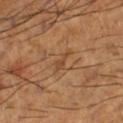Imaged during a routine full-body skin examination; the lesion was not biopsied and no histopathology is available.
Measured at roughly 2.5 mm in maximum diameter.
Located on the left lower leg.
A lesion tile, about 15 mm wide, cut from a 3D total-body photograph.
Automated tile analysis of the lesion measured an area of roughly 2.5 mm² and a shape eccentricity near 0.9. The analysis additionally found a lesion color around L≈46 a*≈21 b*≈34 in CIELAB, a lesion–skin lightness drop of about 7, and a lesion-to-skin contrast of about 5.5 (normalized; higher = more distinct). And it measured a classifier nevus-likeness of about 0/100 and lesion-presence confidence of about 80/100.
Captured under cross-polarized illumination.
A male subject about 65 years old.